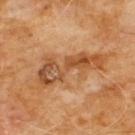follow-up: imaged on a skin check; not biopsied
patient: male, aged around 60
imaging modality: ~15 mm tile from a whole-body skin photo
location: the chest
automated lesion analysis: an area of roughly 17 mm², an eccentricity of roughly 0.95, and a shape-asymmetry score of about 0.45 (0 = symmetric); a lesion color around L≈49 a*≈23 b*≈37 in CIELAB, about 10 CIELAB-L* units darker than the surrounding skin, and a normalized border contrast of about 7.5; a border-irregularity rating of about 7.5/10, a color-variation rating of about 8.5/10, and peripheral color asymmetry of about 2.5
tile lighting: cross-polarized illumination